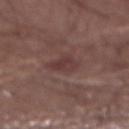<case>
<biopsy_status>not biopsied; imaged during a skin examination</biopsy_status>
<site>abdomen</site>
<patient>
  <sex>male</sex>
  <age_approx>55</age_approx>
</patient>
<automated_metrics>
  <cielab_L>37</cielab_L>
  <cielab_a>20</cielab_a>
  <cielab_b>18</cielab_b>
  <vs_skin_darker_L>7.0</vs_skin_darker_L>
  <nevus_likeness_0_100>0</nevus_likeness_0_100>
  <lesion_detection_confidence_0_100>100</lesion_detection_confidence_0_100>
</automated_metrics>
<lesion_size>
  <long_diameter_mm_approx>2.5</long_diameter_mm_approx>
</lesion_size>
<image>
  <source>total-body photography crop</source>
  <field_of_view_mm>15</field_of_view_mm>
</image>
</case>